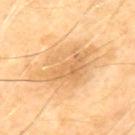Impression:
No biopsy was performed on this lesion — it was imaged during a full skin examination and was not determined to be concerning.
Clinical summary:
A male patient, aged 68 to 72. The tile uses cross-polarized illumination. A 15 mm close-up extracted from a 3D total-body photography capture. An algorithmic analysis of the crop reported border irregularity of about 4.5 on a 0–10 scale and peripheral color asymmetry of about 1.5. From the upper back.